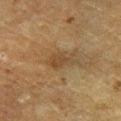<case>
  <biopsy_status>not biopsied; imaged during a skin examination</biopsy_status>
  <lighting>cross-polarized</lighting>
  <site>right lower leg</site>
  <patient>
    <sex>male</sex>
    <age_approx>60</age_approx>
  </patient>
  <image>
    <source>total-body photography crop</source>
    <field_of_view_mm>15</field_of_view_mm>
  </image>
  <automated_metrics>
    <cielab_L>34</cielab_L>
    <cielab_a>13</cielab_a>
    <cielab_b>27</cielab_b>
    <vs_skin_darker_L>6.0</vs_skin_darker_L>
  </automated_metrics>
  <lesion_size>
    <long_diameter_mm_approx>3.5</long_diameter_mm_approx>
  </lesion_size>
</case>